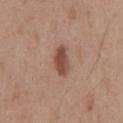The lesion was tiled from a total-body skin photograph and was not biopsied.
The tile uses white-light illumination.
The lesion is located on the front of the torso.
A lesion tile, about 15 mm wide, cut from a 3D total-body photograph.
The patient is a male about 55 years old.